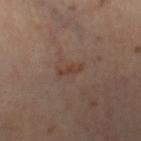Cropped from a whole-body photographic skin survey; the tile spans about 15 mm. Imaged with cross-polarized lighting. The total-body-photography lesion software estimated a footprint of about 2.5 mm², an outline eccentricity of about 0.95 (0 = round, 1 = elongated), and a symmetry-axis asymmetry near 0.3. It also reported border irregularity of about 4 on a 0–10 scale and peripheral color asymmetry of about 0. The analysis additionally found a detector confidence of about 100 out of 100 that the crop contains a lesion. A female subject, aged 33 to 37. Measured at roughly 3 mm in maximum diameter. The lesion is on the right lower leg.